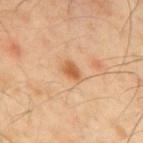Q: Was a biopsy performed?
A: total-body-photography surveillance lesion; no biopsy
Q: What are the patient's age and sex?
A: male, approximately 40 years of age
Q: How large is the lesion?
A: about 2.5 mm
Q: Where on the body is the lesion?
A: the mid back
Q: How was the tile lit?
A: cross-polarized
Q: What kind of image is this?
A: ~15 mm tile from a whole-body skin photo
Q: What did automated image analysis measure?
A: a footprint of about 3.5 mm² and a shape eccentricity near 0.8; an average lesion color of about L≈60 a*≈24 b*≈41 (CIELAB), about 11 CIELAB-L* units darker than the surrounding skin, and a normalized lesion–skin contrast near 8; a border-irregularity rating of about 2/10, a within-lesion color-variation index near 2.5/10, and peripheral color asymmetry of about 1